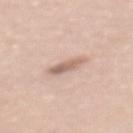Q: Is there a histopathology result?
A: imaged on a skin check; not biopsied
Q: What did automated image analysis measure?
A: a shape eccentricity near 0.9 and a symmetry-axis asymmetry near 0.2; an average lesion color of about L≈63 a*≈18 b*≈26 (CIELAB), about 11 CIELAB-L* units darker than the surrounding skin, and a lesion-to-skin contrast of about 7 (normalized; higher = more distinct)
Q: What is the imaging modality?
A: ~15 mm tile from a whole-body skin photo
Q: How large is the lesion?
A: ~3.5 mm (longest diameter)
Q: Patient demographics?
A: female, aged 58 to 62
Q: Where on the body is the lesion?
A: the mid back
Q: What lighting was used for the tile?
A: white-light illumination